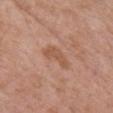Assessment: Imaged during a routine full-body skin examination; the lesion was not biopsied and no histopathology is available. Clinical summary: A female patient, aged around 60. Captured under white-light illumination. On the chest. The lesion's longest dimension is about 4 mm. A roughly 15 mm field-of-view crop from a total-body skin photograph. The total-body-photography lesion software estimated a footprint of about 5 mm² and a symmetry-axis asymmetry near 0.45. The software also gave a nevus-likeness score of about 0/100.